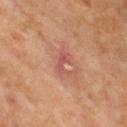Recorded during total-body skin imaging; not selected for excision or biopsy. A female subject in their 50s. A lesion tile, about 15 mm wide, cut from a 3D total-body photograph. Longest diameter approximately 3 mm. The lesion is located on the chest.